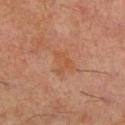– workup: imaged on a skin check; not biopsied
– imaging modality: total-body-photography crop, ~15 mm field of view
– subject: male, aged 58–62
– body site: the right lower leg
– automated lesion analysis: an eccentricity of roughly 0.65 and a shape-asymmetry score of about 0.5 (0 = symmetric); an average lesion color of about L≈49 a*≈23 b*≈33 (CIELAB) and a lesion-to-skin contrast of about 5 (normalized; higher = more distinct)
– tile lighting: cross-polarized
– diameter: about 3 mm